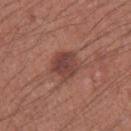This lesion was catalogued during total-body skin photography and was not selected for biopsy.
A 15 mm crop from a total-body photograph taken for skin-cancer surveillance.
The lesion is on the left forearm.
The subject is a male roughly 35 years of age.
The total-body-photography lesion software estimated a footprint of about 8.5 mm² and an eccentricity of roughly 0.55. It also reported a border-irregularity index near 3/10 and radial color variation of about 1.
The tile uses white-light illumination.